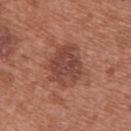notes = imaged on a skin check; not biopsied
subject = female, in their 40s
lighting = white-light
diameter = ≈5 mm
automated lesion analysis = a footprint of about 16 mm², an eccentricity of roughly 0.55, and two-axis asymmetry of about 0.2; an average lesion color of about L≈45 a*≈24 b*≈27 (CIELAB), a lesion–skin lightness drop of about 10, and a normalized border contrast of about 7.5; border irregularity of about 3 on a 0–10 scale, internal color variation of about 3.5 on a 0–10 scale, and radial color variation of about 1
image source = ~15 mm crop, total-body skin-cancer survey
location = the upper back